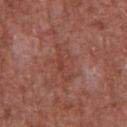Impression:
Captured during whole-body skin photography for melanoma surveillance; the lesion was not biopsied.
Image and clinical context:
Cropped from a whole-body photographic skin survey; the tile spans about 15 mm. The subject is a male in their mid- to late 60s. On the front of the torso. The recorded lesion diameter is about 4.5 mm. This is a white-light tile.